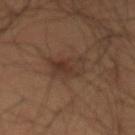Q: Was a biopsy performed?
A: catalogued during a skin exam; not biopsied
Q: How large is the lesion?
A: ≈4.5 mm
Q: What kind of image is this?
A: total-body-photography crop, ~15 mm field of view
Q: Who is the patient?
A: male, about 60 years old
Q: Where on the body is the lesion?
A: the abdomen
Q: What lighting was used for the tile?
A: cross-polarized illumination